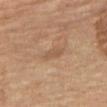Q: Lesion location?
A: the leg
Q: Automated lesion metrics?
A: a lesion color around L≈51 a*≈17 b*≈31 in CIELAB, roughly 7 lightness units darker than nearby skin, and a normalized lesion–skin contrast near 5
Q: How was the tile lit?
A: cross-polarized
Q: Patient demographics?
A: female, in their 70s
Q: How was this image acquired?
A: 15 mm crop, total-body photography
Q: What is the lesion's diameter?
A: about 2.5 mm